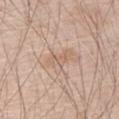The lesion was tiled from a total-body skin photograph and was not biopsied. Automated image analysis of the tile measured an area of roughly 3.5 mm² and a shape-asymmetry score of about 0.4 (0 = symmetric). The software also gave a mean CIELAB color near L≈62 a*≈17 b*≈29, about 7 CIELAB-L* units darker than the surrounding skin, and a normalized lesion–skin contrast near 5. It also reported a classifier nevus-likeness of about 0/100 and a lesion-detection confidence of about 95/100. The lesion's longest dimension is about 3 mm. The lesion is located on the right upper arm. This image is a 15 mm lesion crop taken from a total-body photograph. A male subject aged 68 to 72. Imaged with white-light lighting.